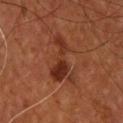- biopsy status — imaged on a skin check; not biopsied
- illumination — cross-polarized illumination
- image-analysis metrics — a classifier nevus-likeness of about 40/100
- lesion size — ~6 mm (longest diameter)
- body site — the back
- patient — male, roughly 55 years of age
- acquisition — ~15 mm crop, total-body skin-cancer survey